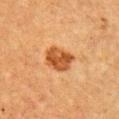{"image": {"source": "total-body photography crop", "field_of_view_mm": 15}, "lighting": "cross-polarized", "patient": {"sex": "male", "age_approx": 50}, "lesion_size": {"long_diameter_mm_approx": 3.5}, "site": "arm", "automated_metrics": {"border_irregularity_0_10": 2.0, "color_variation_0_10": 4.0, "peripheral_color_asymmetry": 1.5, "nevus_likeness_0_100": 85}}